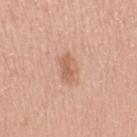follow-up: imaged on a skin check; not biopsied | automated metrics: an eccentricity of roughly 0.85 and two-axis asymmetry of about 0.25; a mean CIELAB color near L≈61 a*≈23 b*≈32 and a lesion-to-skin contrast of about 6.5 (normalized; higher = more distinct); a color-variation rating of about 2.5/10 and radial color variation of about 1 | site: the arm | subject: female, aged around 50 | lesion size: ≈3.5 mm | imaging modality: 15 mm crop, total-body photography | illumination: white-light illumination.